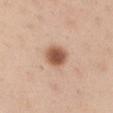| feature | finding |
|---|---|
| follow-up | catalogued during a skin exam; not biopsied |
| imaging modality | 15 mm crop, total-body photography |
| body site | the arm |
| patient | male, about 35 years old |